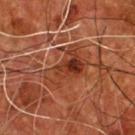Impression: Recorded during total-body skin imaging; not selected for excision or biopsy. Background: Located on the chest. A 15 mm close-up tile from a total-body photography series done for melanoma screening. A male patient, roughly 55 years of age.